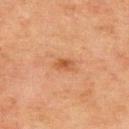The lesion was tiled from a total-body skin photograph and was not biopsied.